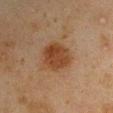Context: The tile uses cross-polarized illumination. Approximately 4 mm at its widest. Located on the right upper arm. A male subject, roughly 45 years of age. A 15 mm close-up extracted from a 3D total-body photography capture.